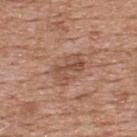Assessment: The lesion was tiled from a total-body skin photograph and was not biopsied. Clinical summary: A male patient, about 60 years old. The tile uses white-light illumination. The lesion's longest dimension is about 4 mm. On the upper back. A 15 mm close-up extracted from a 3D total-body photography capture.